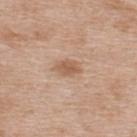Assessment:
Imaged during a routine full-body skin examination; the lesion was not biopsied and no histopathology is available.
Image and clinical context:
The lesion is on the upper back. A 15 mm close-up tile from a total-body photography series done for melanoma screening. The patient is a female aged 38–42. Captured under white-light illumination.